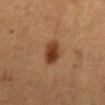follow-up: no biopsy performed (imaged during a skin exam) | tile lighting: cross-polarized illumination | site: the abdomen | patient: female, aged approximately 50 | imaging modality: total-body-photography crop, ~15 mm field of view | automated metrics: a border-irregularity rating of about 1.5/10, a color-variation rating of about 4.5/10, and peripheral color asymmetry of about 1.5.